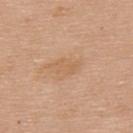lighting = white-light
subject = female, approximately 65 years of age
image-analysis metrics = a footprint of about 4 mm², an eccentricity of roughly 0.8, and a symmetry-axis asymmetry near 0.3; an average lesion color of about L≈61 a*≈20 b*≈35 (CIELAB), a lesion–skin lightness drop of about 6, and a normalized lesion–skin contrast near 4.5; a border-irregularity index near 3/10
lesion size = ~3 mm (longest diameter)
site = the back
acquisition = total-body-photography crop, ~15 mm field of view